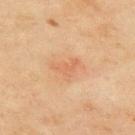Part of a total-body skin-imaging series; this lesion was reviewed on a skin check and was not flagged for biopsy.
Imaged with cross-polarized lighting.
Located on the upper back.
A region of skin cropped from a whole-body photographic capture, roughly 15 mm wide.
A female patient, aged approximately 60.